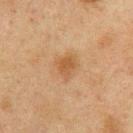Imaged during a routine full-body skin examination; the lesion was not biopsied and no histopathology is available. The lesion is on the chest. This is a cross-polarized tile. Longest diameter approximately 3 mm. A lesion tile, about 15 mm wide, cut from a 3D total-body photograph. A male subject aged 73–77. The total-body-photography lesion software estimated a lesion color around L≈45 a*≈17 b*≈32 in CIELAB and a normalized border contrast of about 6. And it measured a border-irregularity index near 3/10 and a color-variation rating of about 2.5/10. The software also gave an automated nevus-likeness rating near 50 out of 100 and lesion-presence confidence of about 100/100.